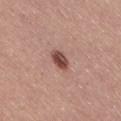{"site": "right thigh", "patient": {"sex": "female", "age_approx": 45}, "image": {"source": "total-body photography crop", "field_of_view_mm": 15}, "lighting": "white-light"}